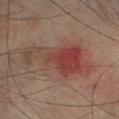No biopsy was performed on this lesion — it was imaged during a full skin examination and was not determined to be concerning. An algorithmic analysis of the crop reported a normalized lesion–skin contrast near 7. And it measured border irregularity of about 6.5 on a 0–10 scale, a within-lesion color-variation index near 8/10, and radial color variation of about 3. And it measured a nevus-likeness score of about 20/100 and a detector confidence of about 100 out of 100 that the crop contains a lesion. From the left lower leg. The patient is a male aged 73 to 77. The tile uses cross-polarized illumination. The lesion's longest dimension is about 9.5 mm. A close-up tile cropped from a whole-body skin photograph, about 15 mm across.